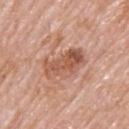Q: Was this lesion biopsied?
A: imaged on a skin check; not biopsied
Q: What kind of image is this?
A: total-body-photography crop, ~15 mm field of view
Q: What are the patient's age and sex?
A: male, in their mid- to late 70s
Q: Where on the body is the lesion?
A: the upper back